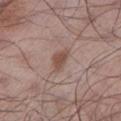| key | value |
|---|---|
| notes | no biopsy performed (imaged during a skin exam) |
| lesion diameter | ≈3 mm |
| image-analysis metrics | a lesion area of about 4.5 mm² and a symmetry-axis asymmetry near 0.35; a border-irregularity index near 3.5/10, a within-lesion color-variation index near 1.5/10, and radial color variation of about 0.5; a nevus-likeness score of about 90/100 and lesion-presence confidence of about 100/100 |
| body site | the leg |
| tile lighting | white-light illumination |
| patient | male, about 55 years old |
| image source | ~15 mm crop, total-body skin-cancer survey |